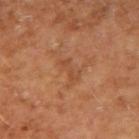This lesion was catalogued during total-body skin photography and was not selected for biopsy.
Measured at roughly 3 mm in maximum diameter.
The subject is a male aged 53–57.
The lesion-visualizer software estimated a lesion area of about 3 mm², an outline eccentricity of about 0.95 (0 = round, 1 = elongated), and a symmetry-axis asymmetry near 0.4. And it measured roughly 6 lightness units darker than nearby skin and a normalized lesion–skin contrast near 5. It also reported border irregularity of about 5.5 on a 0–10 scale, a color-variation rating of about 0/10, and peripheral color asymmetry of about 0. And it measured an automated nevus-likeness rating near 0 out of 100 and a detector confidence of about 100 out of 100 that the crop contains a lesion.
A 15 mm crop from a total-body photograph taken for skin-cancer surveillance.
The lesion is located on the right upper arm.
Imaged with cross-polarized lighting.